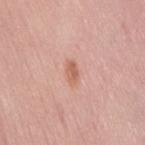Assessment:
The lesion was photographed on a routine skin check and not biopsied; there is no pathology result.
Clinical summary:
Automated tile analysis of the lesion measured a lesion color around L≈62 a*≈24 b*≈31 in CIELAB, about 9 CIELAB-L* units darker than the surrounding skin, and a normalized lesion–skin contrast near 7. And it measured a nevus-likeness score of about 55/100 and a lesion-detection confidence of about 100/100. The lesion's longest dimension is about 2.5 mm. A roughly 15 mm field-of-view crop from a total-body skin photograph. A female subject, aged approximately 50. This is a white-light tile. The lesion is located on the right thigh.